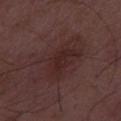Q: How was this image acquired?
A: ~15 mm tile from a whole-body skin photo
Q: Patient demographics?
A: male, about 50 years old